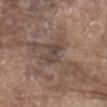No biopsy was performed on this lesion — it was imaged during a full skin examination and was not determined to be concerning. The tile uses white-light illumination. The patient is a male aged approximately 80. From the chest. A lesion tile, about 15 mm wide, cut from a 3D total-body photograph. About 3 mm across.